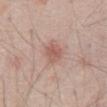Recorded during total-body skin imaging; not selected for excision or biopsy.
From the abdomen.
The total-body-photography lesion software estimated a lesion area of about 5 mm², an eccentricity of roughly 0.65, and a symmetry-axis asymmetry near 0.2. The software also gave a mean CIELAB color near L≈57 a*≈22 b*≈25, about 9 CIELAB-L* units darker than the surrounding skin, and a normalized border contrast of about 6. The software also gave a nevus-likeness score of about 60/100 and lesion-presence confidence of about 100/100.
Measured at roughly 3 mm in maximum diameter.
Cropped from a total-body skin-imaging series; the visible field is about 15 mm.
A male subject roughly 70 years of age.